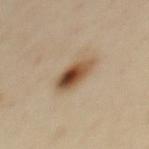{"biopsy_status": "not biopsied; imaged during a skin examination", "site": "upper back", "lighting": "cross-polarized", "image": {"source": "total-body photography crop", "field_of_view_mm": 15}, "lesion_size": {"long_diameter_mm_approx": 4.5}, "patient": {"sex": "female", "age_approx": 40}}